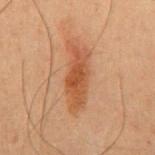acquisition: ~15 mm crop, total-body skin-cancer survey | lesion diameter: ≈7 mm | lighting: cross-polarized illumination | site: the abdomen | image-analysis metrics: an area of roughly 13 mm², a shape eccentricity near 0.95, and a symmetry-axis asymmetry near 0.3; an average lesion color of about L≈39 a*≈20 b*≈29 (CIELAB), about 8 CIELAB-L* units darker than the surrounding skin, and a lesion-to-skin contrast of about 7.5 (normalized; higher = more distinct) | subject: male, in their 50s.